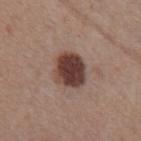Clinical impression: No biopsy was performed on this lesion — it was imaged during a full skin examination and was not determined to be concerning. Background: A 15 mm close-up extracted from a 3D total-body photography capture. The lesion is on the chest. A male subject, aged 53–57. Automated image analysis of the tile measured a lesion area of about 12 mm², a shape eccentricity near 0.5, and a shape-asymmetry score of about 0.15 (0 = symmetric). The software also gave an average lesion color of about L≈39 a*≈19 b*≈22 (CIELAB). The software also gave a classifier nevus-likeness of about 90/100 and a lesion-detection confidence of about 100/100. The recorded lesion diameter is about 4 mm. The tile uses white-light illumination.